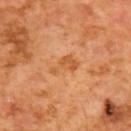Clinical impression:
Part of a total-body skin-imaging series; this lesion was reviewed on a skin check and was not flagged for biopsy.
Image and clinical context:
A 15 mm crop from a total-body photograph taken for skin-cancer surveillance. From the upper back. The subject is a male about 65 years old. The tile uses cross-polarized illumination. The recorded lesion diameter is about 3.5 mm.